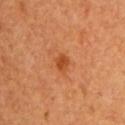Imaged during a routine full-body skin examination; the lesion was not biopsied and no histopathology is available. A 15 mm crop from a total-body photograph taken for skin-cancer surveillance. The patient is a female aged 48 to 52. Captured under cross-polarized illumination. Measured at roughly 2 mm in maximum diameter. The lesion is located on the right upper arm.